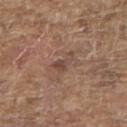This lesion was catalogued during total-body skin photography and was not selected for biopsy. The subject is a male aged around 80. Imaged with white-light lighting. The lesion is located on the upper back. Longest diameter approximately 3 mm. A lesion tile, about 15 mm wide, cut from a 3D total-body photograph.